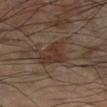Impression:
Part of a total-body skin-imaging series; this lesion was reviewed on a skin check and was not flagged for biopsy.
Acquisition and patient details:
A male patient, approximately 65 years of age. The recorded lesion diameter is about 5 mm. Captured under cross-polarized illumination. Cropped from a whole-body photographic skin survey; the tile spans about 15 mm. The lesion is on the left forearm. Automated tile analysis of the lesion measured a lesion color around L≈34 a*≈16 b*≈23 in CIELAB, a lesion–skin lightness drop of about 7, and a normalized border contrast of about 7.5. And it measured internal color variation of about 3.5 on a 0–10 scale and a peripheral color-asymmetry measure near 1.